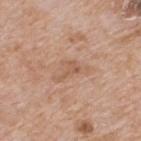workup — no biopsy performed (imaged during a skin exam); image — total-body-photography crop, ~15 mm field of view; diameter — ≈3 mm; patient — male, aged approximately 65; anatomic site — the back; illumination — white-light illumination.